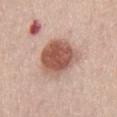The lesion was photographed on a routine skin check and not biopsied; there is no pathology result.
The lesion's longest dimension is about 5 mm.
Imaged with white-light lighting.
The lesion is on the chest.
The patient is a male aged 53 to 57.
A roughly 15 mm field-of-view crop from a total-body skin photograph.